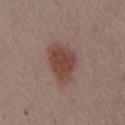Impression:
Captured during whole-body skin photography for melanoma surveillance; the lesion was not biopsied.
Acquisition and patient details:
Measured at roughly 5 mm in maximum diameter. A male subject aged approximately 55. The lesion is located on the chest. A region of skin cropped from a whole-body photographic capture, roughly 15 mm wide. An algorithmic analysis of the crop reported a lesion area of about 13 mm², an eccentricity of roughly 0.7, and a shape-asymmetry score of about 0.25 (0 = symmetric). It also reported an average lesion color of about L≈44 a*≈20 b*≈24 (CIELAB), a lesion–skin lightness drop of about 10, and a lesion-to-skin contrast of about 9 (normalized; higher = more distinct). The analysis additionally found a classifier nevus-likeness of about 100/100 and lesion-presence confidence of about 100/100. Imaged with white-light lighting.